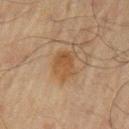This lesion was catalogued during total-body skin photography and was not selected for biopsy. About 3.5 mm across. A male patient, roughly 70 years of age. A region of skin cropped from a whole-body photographic capture, roughly 15 mm wide. The tile uses cross-polarized illumination. The lesion is on the left upper arm.